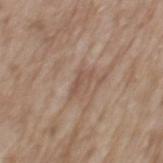<lesion>
<biopsy_status>not biopsied; imaged during a skin examination</biopsy_status>
<patient>
  <sex>male</sex>
  <age_approx>70</age_approx>
</patient>
<image>
  <source>total-body photography crop</source>
  <field_of_view_mm>15</field_of_view_mm>
</image>
<lesion_size>
  <long_diameter_mm_approx>3.0</long_diameter_mm_approx>
</lesion_size>
<site>mid back</site>
<automated_metrics>
  <cielab_L>52</cielab_L>
  <cielab_a>18</cielab_a>
  <cielab_b>26</cielab_b>
  <vs_skin_darker_L>7.0</vs_skin_darker_L>
  <color_variation_0_10>0.5</color_variation_0_10>
  <peripheral_color_asymmetry>0.0</peripheral_color_asymmetry>
</automated_metrics>
</lesion>